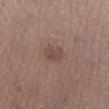Q: What is the anatomic site?
A: the right lower leg
Q: How was the tile lit?
A: white-light
Q: Automated lesion metrics?
A: a lesion color around L≈47 a*≈18 b*≈24 in CIELAB, roughly 7 lightness units darker than nearby skin, and a normalized border contrast of about 5.5; an automated nevus-likeness rating near 15 out of 100
Q: What is the imaging modality?
A: total-body-photography crop, ~15 mm field of view
Q: Patient demographics?
A: male, aged 53 to 57